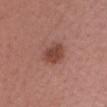<record>
<biopsy_status>not biopsied; imaged during a skin examination</biopsy_status>
<image>
  <source>total-body photography crop</source>
  <field_of_view_mm>15</field_of_view_mm>
</image>
<site>head or neck</site>
<lesion_size>
  <long_diameter_mm_approx>3.5</long_diameter_mm_approx>
</lesion_size>
<patient>
  <sex>female</sex>
  <age_approx>55</age_approx>
</patient>
<lighting>white-light</lighting>
</record>